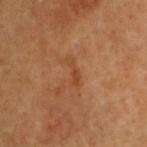| key | value |
|---|---|
| body site | the head or neck |
| acquisition | total-body-photography crop, ~15 mm field of view |
| subject | male, aged around 60 |
| lesion diameter | about 3.5 mm |